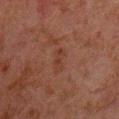Impression: This lesion was catalogued during total-body skin photography and was not selected for biopsy. Context: On the chest. Imaged with cross-polarized lighting. A male subject in their 60s. The lesion-visualizer software estimated an eccentricity of roughly 0.85 and two-axis asymmetry of about 0.3. The analysis additionally found a border-irregularity index near 3.5/10 and radial color variation of about 0.5. A roughly 15 mm field-of-view crop from a total-body skin photograph. About 3 mm across.